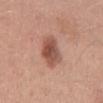biopsy status — total-body-photography surveillance lesion; no biopsy | imaging modality — ~15 mm crop, total-body skin-cancer survey | patient — male, aged approximately 30 | size — ≈5.5 mm | automated lesion analysis — an area of roughly 11 mm², an eccentricity of roughly 0.9, and a shape-asymmetry score of about 0.2 (0 = symmetric); border irregularity of about 2.5 on a 0–10 scale, a color-variation rating of about 4/10, and peripheral color asymmetry of about 1; a nevus-likeness score of about 60/100 and a detector confidence of about 100 out of 100 that the crop contains a lesion | location — the mid back | tile lighting — white-light illumination.